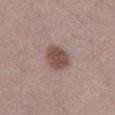{"biopsy_status": "not biopsied; imaged during a skin examination", "patient": {"sex": "female", "age_approx": 40}, "lighting": "white-light", "image": {"source": "total-body photography crop", "field_of_view_mm": 15}, "site": "mid back", "lesion_size": {"long_diameter_mm_approx": 4.0}}